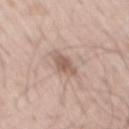Q: Is there a histopathology result?
A: imaged on a skin check; not biopsied
Q: What are the patient's age and sex?
A: male, aged around 50
Q: What is the imaging modality?
A: ~15 mm tile from a whole-body skin photo
Q: How large is the lesion?
A: ≈3.5 mm
Q: Lesion location?
A: the mid back